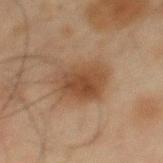Q: Who is the patient?
A: male, about 45 years old
Q: Lesion location?
A: the back
Q: Lesion size?
A: ~5 mm (longest diameter)
Q: Illumination type?
A: cross-polarized
Q: How was this image acquired?
A: total-body-photography crop, ~15 mm field of view